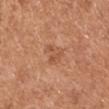| field | value |
|---|---|
| workup | no biopsy performed (imaged during a skin exam) |
| patient | male, about 55 years old |
| image source | 15 mm crop, total-body photography |
| body site | the chest |
| lighting | white-light |
| image-analysis metrics | a border-irregularity index near 3.5/10, internal color variation of about 2.5 on a 0–10 scale, and radial color variation of about 1; a classifier nevus-likeness of about 0/100 and a lesion-detection confidence of about 100/100 |